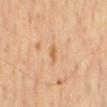{
  "automated_metrics": {
    "area_mm2_approx": 2.0,
    "vs_skin_contrast_norm": 6.5
  },
  "patient": {
    "sex": "male",
    "age_approx": 65
  },
  "lighting": "cross-polarized",
  "image": {
    "source": "total-body photography crop",
    "field_of_view_mm": 15
  },
  "site": "back"
}